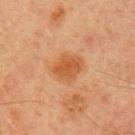The lesion was tiled from a total-body skin photograph and was not biopsied. Captured under cross-polarized illumination. Automated image analysis of the tile measured an average lesion color of about L≈45 a*≈22 b*≈35 (CIELAB) and a normalized border contrast of about 7. And it measured a lesion-detection confidence of about 100/100. The lesion is on the arm. Cropped from a total-body skin-imaging series; the visible field is about 15 mm. A male subject, roughly 70 years of age.